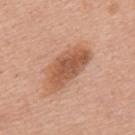{
  "biopsy_status": "not biopsied; imaged during a skin examination",
  "patient": {
    "sex": "male",
    "age_approx": 55
  },
  "lesion_size": {
    "long_diameter_mm_approx": 6.5
  },
  "image": {
    "source": "total-body photography crop",
    "field_of_view_mm": 15
  },
  "site": "mid back",
  "lighting": "white-light"
}